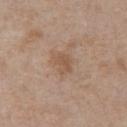acquisition: total-body-photography crop, ~15 mm field of view | lesion diameter: about 2.5 mm | image-analysis metrics: a lesion area of about 4.5 mm², a shape eccentricity near 0.6, and two-axis asymmetry of about 0.35 | subject: male, aged approximately 65 | lighting: white-light illumination | body site: the chest.